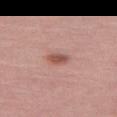{
  "biopsy_status": "not biopsied; imaged during a skin examination",
  "patient": {
    "sex": "female",
    "age_approx": 70
  },
  "image": {
    "source": "total-body photography crop",
    "field_of_view_mm": 15
  },
  "site": "left thigh"
}